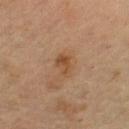Acquisition and patient details: Imaged with cross-polarized lighting. The total-body-photography lesion software estimated a nevus-likeness score of about 15/100. A female patient, in their mid- to late 50s. Longest diameter approximately 3 mm. Located on the right lower leg. A close-up tile cropped from a whole-body skin photograph, about 15 mm across.